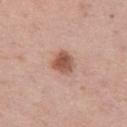No biopsy was performed on this lesion — it was imaged during a full skin examination and was not determined to be concerning. The subject is a female about 40 years old. The recorded lesion diameter is about 3 mm. A close-up tile cropped from a whole-body skin photograph, about 15 mm across. The lesion-visualizer software estimated a border-irregularity rating of about 2/10. The software also gave lesion-presence confidence of about 100/100. The lesion is on the left thigh.